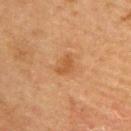workup: no biopsy performed (imaged during a skin exam) | imaging modality: 15 mm crop, total-body photography | diameter: ≈3 mm | site: the upper back | subject: male, aged approximately 60.